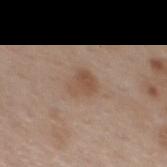Case summary:
• follow-up · imaged on a skin check; not biopsied
• image source · ~15 mm crop, total-body skin-cancer survey
• lighting · white-light illumination
• anatomic site · the mid back
• diameter · ~3 mm (longest diameter)
• subject · female, approximately 40 years of age